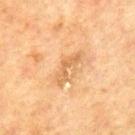Clinical impression: The lesion was photographed on a routine skin check and not biopsied; there is no pathology result. Background: The recorded lesion diameter is about 4.5 mm. Automated tile analysis of the lesion measured a classifier nevus-likeness of about 0/100 and lesion-presence confidence of about 100/100. The subject is a male in their 70s. The tile uses cross-polarized illumination. A close-up tile cropped from a whole-body skin photograph, about 15 mm across. Located on the mid back.